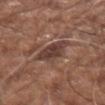biopsy status: catalogued during a skin exam; not biopsied | location: the right upper arm | lesion size: ~3.5 mm (longest diameter) | patient: male, in their mid- to late 70s | illumination: white-light illumination | image source: ~15 mm crop, total-body skin-cancer survey.